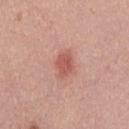This lesion was catalogued during total-body skin photography and was not selected for biopsy.
A 15 mm close-up extracted from a 3D total-body photography capture.
A male patient, aged 28–32.
This is a white-light tile.
The lesion-visualizer software estimated an eccentricity of roughly 0.7 and a symmetry-axis asymmetry near 0.15. It also reported an average lesion color of about L≈56 a*≈28 b*≈27 (CIELAB), about 10 CIELAB-L* units darker than the surrounding skin, and a lesion-to-skin contrast of about 7 (normalized; higher = more distinct). It also reported a color-variation rating of about 2/10 and a peripheral color-asymmetry measure near 1.
About 3 mm across.